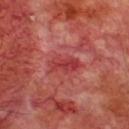This lesion was catalogued during total-body skin photography and was not selected for biopsy. A 15 mm close-up tile from a total-body photography series done for melanoma screening. Located on the chest. The subject is a male aged around 70.